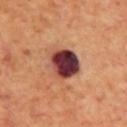workup: imaged on a skin check; not biopsied
location: the upper back
subject: male, aged approximately 70
image source: 15 mm crop, total-body photography